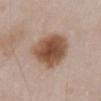Assessment:
The lesion was photographed on a routine skin check and not biopsied; there is no pathology result.
Image and clinical context:
Imaged with white-light lighting. Approximately 5.5 mm at its widest. Located on the front of the torso. A 15 mm close-up tile from a total-body photography series done for melanoma screening. A male patient aged 53–57.